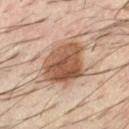Q: Was a biopsy performed?
A: imaged on a skin check; not biopsied
Q: What did automated image analysis measure?
A: a within-lesion color-variation index near 6/10 and radial color variation of about 2
Q: What is the anatomic site?
A: the left forearm
Q: Who is the patient?
A: male, aged approximately 40
Q: What lighting was used for the tile?
A: cross-polarized illumination
Q: Lesion size?
A: ≈6 mm
Q: What kind of image is this?
A: ~15 mm crop, total-body skin-cancer survey